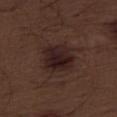follow-up: catalogued during a skin exam; not biopsied
lighting: white-light illumination
size: ≈4.5 mm
TBP lesion metrics: a lesion area of about 15 mm² and a shape eccentricity near 0.55; an average lesion color of about L≈21 a*≈16 b*≈16 (CIELAB), about 8 CIELAB-L* units darker than the surrounding skin, and a lesion-to-skin contrast of about 10 (normalized; higher = more distinct); internal color variation of about 4.5 on a 0–10 scale and peripheral color asymmetry of about 1.5; an automated nevus-likeness rating near 85 out of 100
acquisition: 15 mm crop, total-body photography
location: the right thigh
patient: male, aged approximately 70